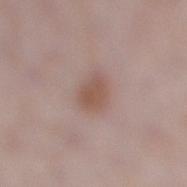Clinical impression: Part of a total-body skin-imaging series; this lesion was reviewed on a skin check and was not flagged for biopsy. Clinical summary: A female subject roughly 30 years of age. The lesion's longest dimension is about 3 mm. Captured under white-light illumination. The lesion-visualizer software estimated a footprint of about 5.5 mm², an eccentricity of roughly 0.65, and a shape-asymmetry score of about 0.2 (0 = symmetric). The analysis additionally found a lesion color around L≈53 a*≈18 b*≈25 in CIELAB and a normalized border contrast of about 7.5. The software also gave a classifier nevus-likeness of about 95/100. Cropped from a total-body skin-imaging series; the visible field is about 15 mm. On the leg.